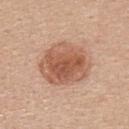notes = total-body-photography surveillance lesion; no biopsy | body site = the upper back | illumination = white-light illumination | imaging modality = 15 mm crop, total-body photography | automated metrics = a lesion area of about 24 mm², a shape eccentricity near 0.55, and a symmetry-axis asymmetry near 0.15; an average lesion color of about L≈57 a*≈23 b*≈31 (CIELAB), roughly 12 lightness units darker than nearby skin, and a normalized lesion–skin contrast near 8; a border-irregularity index near 1.5/10, a color-variation rating of about 5.5/10, and a peripheral color-asymmetry measure near 1.5 | patient = female, aged 33–37 | size = ≈6 mm.